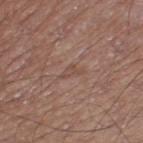Q: Was this lesion biopsied?
A: catalogued during a skin exam; not biopsied
Q: What is the imaging modality?
A: 15 mm crop, total-body photography
Q: Where on the body is the lesion?
A: the left thigh
Q: What are the patient's age and sex?
A: male, aged approximately 55